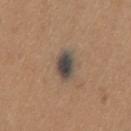<case>
<biopsy_status>not biopsied; imaged during a skin examination</biopsy_status>
<site>chest</site>
<patient>
  <sex>male</sex>
  <age_approx>65</age_approx>
</patient>
<image>
  <source>total-body photography crop</source>
  <field_of_view_mm>15</field_of_view_mm>
</image>
</case>